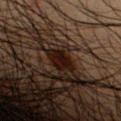No biopsy was performed on this lesion — it was imaged during a full skin examination and was not determined to be concerning. On the right forearm. A roughly 15 mm field-of-view crop from a total-body skin photograph. The patient is a male in their 50s. The recorded lesion diameter is about 3 mm. Captured under cross-polarized illumination.